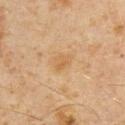Q: Was a biopsy performed?
A: no biopsy performed (imaged during a skin exam)
Q: What is the anatomic site?
A: the chest
Q: What is the lesion's diameter?
A: about 2.5 mm
Q: What is the imaging modality?
A: total-body-photography crop, ~15 mm field of view
Q: Illumination type?
A: cross-polarized illumination
Q: Who is the patient?
A: male, aged 58 to 62